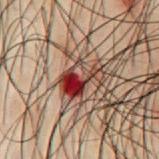Captured during whole-body skin photography for melanoma surveillance; the lesion was not biopsied.
A male patient, roughly 50 years of age.
On the chest.
Automated image analysis of the tile measured a footprint of about 9.5 mm², an eccentricity of roughly 0.75, and a symmetry-axis asymmetry near 0.4. The software also gave an average lesion color of about L≈30 a*≈22 b*≈22 (CIELAB), about 13 CIELAB-L* units darker than the surrounding skin, and a normalized border contrast of about 12. And it measured a classifier nevus-likeness of about 0/100 and lesion-presence confidence of about 100/100.
About 4.5 mm across.
This is a cross-polarized tile.
A 15 mm close-up extracted from a 3D total-body photography capture.